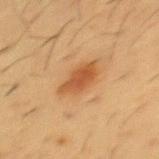biopsy status: catalogued during a skin exam; not biopsied | anatomic site: the chest | patient: male, aged approximately 55 | image: total-body-photography crop, ~15 mm field of view | lesion size: ≈4.5 mm.